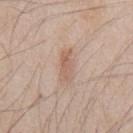Captured during whole-body skin photography for melanoma surveillance; the lesion was not biopsied.
From the chest.
This is a white-light tile.
Measured at roughly 4 mm in maximum diameter.
The subject is a male roughly 45 years of age.
A 15 mm close-up tile from a total-body photography series done for melanoma screening.
The lesion-visualizer software estimated a color-variation rating of about 3.5/10 and a peripheral color-asymmetry measure near 1.